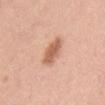| feature | finding |
|---|---|
| notes | total-body-photography surveillance lesion; no biopsy |
| location | the front of the torso |
| subject | male, approximately 55 years of age |
| acquisition | total-body-photography crop, ~15 mm field of view |